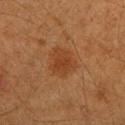The lesion was photographed on a routine skin check and not biopsied; there is no pathology result. Located on the arm. Automated tile analysis of the lesion measured an area of roughly 8.5 mm², an eccentricity of roughly 0.3, and a symmetry-axis asymmetry near 0.25. A female subject, in their 40s. Cropped from a total-body skin-imaging series; the visible field is about 15 mm.